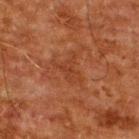This lesion was catalogued during total-body skin photography and was not selected for biopsy.
The lesion's longest dimension is about 3.5 mm.
A region of skin cropped from a whole-body photographic capture, roughly 15 mm wide.
On the upper back.
The total-body-photography lesion software estimated a lesion-to-skin contrast of about 5 (normalized; higher = more distinct). The analysis additionally found a classifier nevus-likeness of about 0/100.
A male patient, about 60 years old.
Captured under cross-polarized illumination.